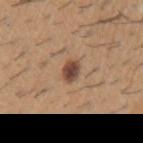{"biopsy_status": "not biopsied; imaged during a skin examination", "image": {"source": "total-body photography crop", "field_of_view_mm": 15}, "patient": {"sex": "male", "age_approx": 60}, "lesion_size": {"long_diameter_mm_approx": 2.5}, "site": "left upper arm", "lighting": "white-light"}